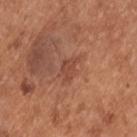<case>
  <biopsy_status>not biopsied; imaged during a skin examination</biopsy_status>
  <site>upper back</site>
  <automated_metrics>
    <area_mm2_approx>4.5</area_mm2_approx>
    <eccentricity>0.75</eccentricity>
    <shape_asymmetry>0.4</shape_asymmetry>
    <color_variation_0_10>1.5</color_variation_0_10>
    <peripheral_color_asymmetry>0.5</peripheral_color_asymmetry>
    <nevus_likeness_0_100>0</nevus_likeness_0_100>
    <lesion_detection_confidence_0_100>100</lesion_detection_confidence_0_100>
  </automated_metrics>
  <patient>
    <sex>male</sex>
    <age_approx>55</age_approx>
  </patient>
  <image>
    <source>total-body photography crop</source>
    <field_of_view_mm>15</field_of_view_mm>
  </image>
</case>